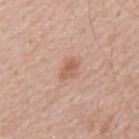No biopsy was performed on this lesion — it was imaged during a full skin examination and was not determined to be concerning.
A 15 mm crop from a total-body photograph taken for skin-cancer surveillance.
The lesion is located on the chest.
The patient is a male aged 58–62.